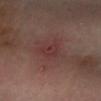| key | value |
|---|---|
| image source | ~15 mm crop, total-body skin-cancer survey |
| lesion diameter | ≈2.5 mm |
| subject | male, aged 53–57 |
| illumination | cross-polarized illumination |
| body site | the left forearm |